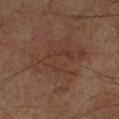Q: Was a biopsy performed?
A: no biopsy performed (imaged during a skin exam)
Q: Illumination type?
A: cross-polarized illumination
Q: What is the anatomic site?
A: the left lower leg
Q: What kind of image is this?
A: ~15 mm tile from a whole-body skin photo
Q: Patient demographics?
A: male, in their mid- to late 60s
Q: Automated lesion metrics?
A: a mean CIELAB color near L≈36 a*≈17 b*≈25, about 5 CIELAB-L* units darker than the surrounding skin, and a normalized lesion–skin contrast near 5; a border-irregularity index near 8/10, internal color variation of about 3 on a 0–10 scale, and peripheral color asymmetry of about 1